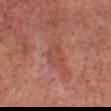| feature | finding |
|---|---|
| workup | imaged on a skin check; not biopsied |
| size | about 2.5 mm |
| subject | male, about 55 years old |
| lighting | cross-polarized illumination |
| anatomic site | the head or neck |
| image source | ~15 mm tile from a whole-body skin photo |